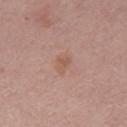Assessment: The lesion was tiled from a total-body skin photograph and was not biopsied. Clinical summary: Imaged with white-light lighting. Automated tile analysis of the lesion measured an outline eccentricity of about 0.8 (0 = round, 1 = elongated) and two-axis asymmetry of about 0.3. The software also gave an average lesion color of about L≈56 a*≈20 b*≈27 (CIELAB) and a lesion-to-skin contrast of about 5 (normalized; higher = more distinct). The lesion is on the right thigh. A close-up tile cropped from a whole-body skin photograph, about 15 mm across. A female subject, in their mid- to late 50s.